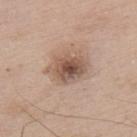Recorded during total-body skin imaging; not selected for excision or biopsy. About 5.5 mm across. A 15 mm close-up extracted from a 3D total-body photography capture. A male subject aged approximately 65. From the upper back. The tile uses white-light illumination.